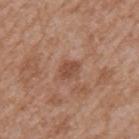biopsy status=total-body-photography surveillance lesion; no biopsy
imaging modality=~15 mm crop, total-body skin-cancer survey
body site=the mid back
subject=male, in their mid- to late 60s